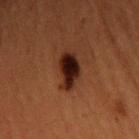<case>
<biopsy_status>not biopsied; imaged during a skin examination</biopsy_status>
<patient>
  <sex>male</sex>
  <age_approx>50</age_approx>
</patient>
<site>chest</site>
<image>
  <source>total-body photography crop</source>
  <field_of_view_mm>15</field_of_view_mm>
</image>
</case>